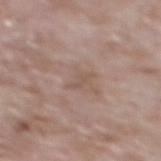| field | value |
|---|---|
| biopsy status | total-body-photography surveillance lesion; no biopsy |
| automated metrics | an area of roughly 3 mm², a shape eccentricity near 0.9, and two-axis asymmetry of about 0.4; a lesion color around L≈53 a*≈16 b*≈24 in CIELAB, a lesion–skin lightness drop of about 6, and a normalized border contrast of about 4.5 |
| diameter | ≈3 mm |
| site | the back |
| image | 15 mm crop, total-body photography |
| patient | male, in their 60s |
| tile lighting | white-light illumination |